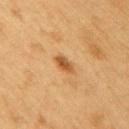Findings:
- follow-up · catalogued during a skin exam; not biopsied
- acquisition · ~15 mm tile from a whole-body skin photo
- illumination · cross-polarized
- patient · male, in their mid-50s
- body site · the right upper arm
- automated lesion analysis · a footprint of about 4 mm², a shape eccentricity near 0.75, and a shape-asymmetry score of about 0.25 (0 = symmetric); an average lesion color of about L≈49 a*≈21 b*≈38 (CIELAB) and a normalized border contrast of about 8; a border-irregularity index near 2.5/10, internal color variation of about 3.5 on a 0–10 scale, and a peripheral color-asymmetry measure near 1